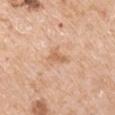<record>
  <biopsy_status>not biopsied; imaged during a skin examination</biopsy_status>
  <lesion_size>
    <long_diameter_mm_approx>2.5</long_diameter_mm_approx>
  </lesion_size>
  <site>arm</site>
  <automated_metrics>
    <border_irregularity_0_10>4.5</border_irregularity_0_10>
    <color_variation_0_10>1.5</color_variation_0_10>
    <peripheral_color_asymmetry>0.5</peripheral_color_asymmetry>
    <nevus_likeness_0_100>0</nevus_likeness_0_100>
    <lesion_detection_confidence_0_100>100</lesion_detection_confidence_0_100>
  </automated_metrics>
  <image>
    <source>total-body photography crop</source>
    <field_of_view_mm>15</field_of_view_mm>
  </image>
  <patient>
    <sex>male</sex>
    <age_approx>65</age_approx>
  </patient>
  <lighting>white-light</lighting>
</record>